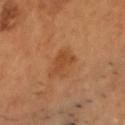biopsy_status: not biopsied; imaged during a skin examination
image:
  source: total-body photography crop
  field_of_view_mm: 15
lighting: cross-polarized
automated_metrics:
  cielab_L: 46
  cielab_a: 24
  cielab_b: 38
  vs_skin_darker_L: 7.0
  vs_skin_contrast_norm: 6.0
  border_irregularity_0_10: 4.0
  color_variation_0_10: 2.0
  peripheral_color_asymmetry: 0.5
site: head or neck
patient:
  sex: male
  age_approx: 45